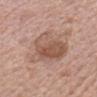lighting: white-light
automated_metrics:
  area_mm2_approx: 19.0
  eccentricity: 0.7
  cielab_L: 54
  cielab_a: 19
  cielab_b: 27
  vs_skin_darker_L: 10.0
  vs_skin_contrast_norm: 7.0
  nevus_likeness_0_100: 15
  lesion_detection_confidence_0_100: 100
patient:
  sex: male
  age_approx: 45
site: head or neck
image:
  source: total-body photography crop
  field_of_view_mm: 15